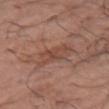No biopsy was performed on this lesion — it was imaged during a full skin examination and was not determined to be concerning. The subject is a male aged approximately 70. Located on the arm. Automated image analysis of the tile measured a footprint of about 8.5 mm², an outline eccentricity of about 0.7 (0 = round, 1 = elongated), and a symmetry-axis asymmetry near 0.3. It also reported a mean CIELAB color near L≈47 a*≈21 b*≈27, a lesion–skin lightness drop of about 6, and a normalized border contrast of about 5. It also reported a classifier nevus-likeness of about 0/100. A roughly 15 mm field-of-view crop from a total-body skin photograph. Imaged with white-light lighting.